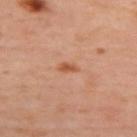Part of a total-body skin-imaging series; this lesion was reviewed on a skin check and was not flagged for biopsy.
This is a cross-polarized tile.
The lesion is on the upper back.
A female subject, approximately 40 years of age.
This image is a 15 mm lesion crop taken from a total-body photograph.
Longest diameter approximately 2.5 mm.